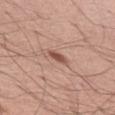Imaged during a routine full-body skin examination; the lesion was not biopsied and no histopathology is available.
A male subject approximately 55 years of age.
About 2.5 mm across.
A 15 mm close-up extracted from a 3D total-body photography capture.
An algorithmic analysis of the crop reported an average lesion color of about L≈51 a*≈23 b*≈27 (CIELAB) and a normalized lesion–skin contrast near 9. The software also gave border irregularity of about 2.5 on a 0–10 scale, internal color variation of about 1 on a 0–10 scale, and radial color variation of about 0.5.